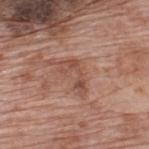Captured during whole-body skin photography for melanoma surveillance; the lesion was not biopsied. From the upper back. A male patient, aged 68–72. Imaged with white-light lighting. The lesion-visualizer software estimated a mean CIELAB color near L≈49 a*≈21 b*≈28, roughly 9 lightness units darker than nearby skin, and a normalized lesion–skin contrast near 6.5. About 4 mm across. A 15 mm crop from a total-body photograph taken for skin-cancer surveillance.